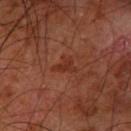follow-up — total-body-photography surveillance lesion; no biopsy | subject — male, roughly 60 years of age | image — 15 mm crop, total-body photography | lesion diameter — about 3 mm | anatomic site — the arm | tile lighting — cross-polarized.